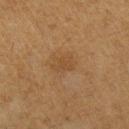Imaged during a routine full-body skin examination; the lesion was not biopsied and no histopathology is available.
A roughly 15 mm field-of-view crop from a total-body skin photograph.
A female patient, in their mid-50s.
On the left upper arm.